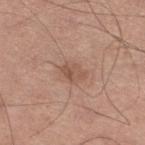Impression:
Recorded during total-body skin imaging; not selected for excision or biopsy.
Background:
A lesion tile, about 15 mm wide, cut from a 3D total-body photograph. Measured at roughly 2.5 mm in maximum diameter. A male patient aged 53–57. Located on the left lower leg.